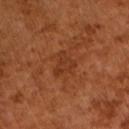The lesion's longest dimension is about 3.5 mm. A 15 mm close-up tile from a total-body photography series done for melanoma screening. Captured under cross-polarized illumination. The lesion-visualizer software estimated an average lesion color of about L≈35 a*≈25 b*≈34 (CIELAB). It also reported a border-irregularity index near 5.5/10 and peripheral color asymmetry of about 0.5. It also reported a classifier nevus-likeness of about 0/100 and lesion-presence confidence of about 100/100. A male patient roughly 65 years of age.